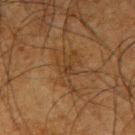<tbp_lesion>
  <biopsy_status>not biopsied; imaged during a skin examination</biopsy_status>
  <patient>
    <sex>male</sex>
    <age_approx>65</age_approx>
  </patient>
  <automated_metrics>
    <border_irregularity_0_10>8.0</border_irregularity_0_10>
    <color_variation_0_10>3.0</color_variation_0_10>
    <peripheral_color_asymmetry>1.0</peripheral_color_asymmetry>
    <nevus_likeness_0_100>0</nevus_likeness_0_100>
    <lesion_detection_confidence_0_100>60</lesion_detection_confidence_0_100>
  </automated_metrics>
  <lesion_size>
    <long_diameter_mm_approx>6.0</long_diameter_mm_approx>
  </lesion_size>
  <lighting>cross-polarized</lighting>
  <site>right upper arm</site>
  <image>
    <source>total-body photography crop</source>
    <field_of_view_mm>15</field_of_view_mm>
  </image>
</tbp_lesion>